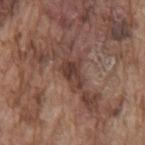The lesion was tiled from a total-body skin photograph and was not biopsied. The total-body-photography lesion software estimated a footprint of about 5 mm² and two-axis asymmetry of about 0.4. It also reported a lesion color around L≈38 a*≈19 b*≈23 in CIELAB, a lesion–skin lightness drop of about 10, and a lesion-to-skin contrast of about 8.5 (normalized; higher = more distinct). A 15 mm crop from a total-body photograph taken for skin-cancer surveillance. Located on the mid back. A male patient approximately 75 years of age. The recorded lesion diameter is about 3 mm.